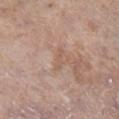Clinical impression: The lesion was photographed on a routine skin check and not biopsied; there is no pathology result. Image and clinical context: The recorded lesion diameter is about 3 mm. From the leg. The tile uses white-light illumination. A female patient aged approximately 85 or older. The total-body-photography lesion software estimated a lesion area of about 2.5 mm². The software also gave a lesion color around L≈57 a*≈17 b*≈28 in CIELAB, a lesion–skin lightness drop of about 6, and a lesion-to-skin contrast of about 5 (normalized; higher = more distinct). And it measured a lesion-detection confidence of about 100/100. This image is a 15 mm lesion crop taken from a total-body photograph.